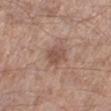<case>
  <biopsy_status>not biopsied; imaged during a skin examination</biopsy_status>
  <patient>
    <sex>male</sex>
    <age_approx>55</age_approx>
  </patient>
  <lighting>white-light</lighting>
  <image>
    <source>total-body photography crop</source>
    <field_of_view_mm>15</field_of_view_mm>
  </image>
  <site>left lower leg</site>
  <lesion_size>
    <long_diameter_mm_approx>2.5</long_diameter_mm_approx>
  </lesion_size>
</case>